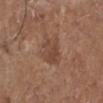Recorded during total-body skin imaging; not selected for excision or biopsy. A male patient in their mid- to late 70s. A 15 mm close-up tile from a total-body photography series done for melanoma screening. This is a white-light tile. Located on the left lower leg.